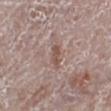Recorded during total-body skin imaging; not selected for excision or biopsy. This image is a 15 mm lesion crop taken from a total-body photograph. A male patient aged approximately 70. The lesion is on the leg. This is a white-light tile. The recorded lesion diameter is about 2.5 mm.